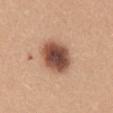notes=imaged on a skin check; not biopsied | imaging modality=~15 mm tile from a whole-body skin photo | lighting=white-light illumination | image-analysis metrics=an area of roughly 13 mm²; a border-irregularity rating of about 1.5/10, internal color variation of about 6.5 on a 0–10 scale, and a peripheral color-asymmetry measure near 1.5 | diameter=~4.5 mm (longest diameter) | site=the mid back | subject=female, aged 28 to 32.